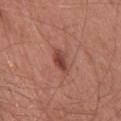  biopsy_status: not biopsied; imaged during a skin examination
  lighting: white-light
  site: head or neck
  automated_metrics:
    eccentricity: 0.85
    shape_asymmetry: 0.25
    border_irregularity_0_10: 2.5
    color_variation_0_10: 4.0
    peripheral_color_asymmetry: 1.5
    nevus_likeness_0_100: 55
    lesion_detection_confidence_0_100: 100
  image:
    source: total-body photography crop
    field_of_view_mm: 15
  patient:
    sex: female
    age_approx: 65